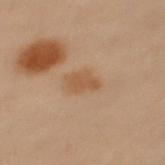notes=no biopsy performed (imaged during a skin exam) | imaging modality=~15 mm crop, total-body skin-cancer survey | site=the upper back | subject=female, approximately 60 years of age.